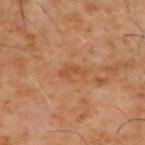image:
  source: total-body photography crop
  field_of_view_mm: 15
lesion_size:
  long_diameter_mm_approx: 3.0
lighting: cross-polarized
patient:
  sex: male
  age_approx: 60
site: upper back